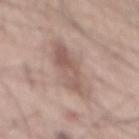Q: Was a biopsy performed?
A: total-body-photography surveillance lesion; no biopsy
Q: What kind of image is this?
A: ~15 mm tile from a whole-body skin photo
Q: Who is the patient?
A: male, aged 53 to 57
Q: Where on the body is the lesion?
A: the back